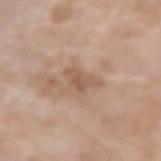This lesion was catalogued during total-body skin photography and was not selected for biopsy. A female patient aged 73 to 77. Measured at roughly 3.5 mm in maximum diameter. Located on the right forearm. A 15 mm crop from a total-body photograph taken for skin-cancer surveillance. Imaged with white-light lighting. An algorithmic analysis of the crop reported a normalized lesion–skin contrast near 6.